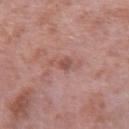notes=imaged on a skin check; not biopsied
patient=male, roughly 40 years of age
lesion size=≈4 mm
illumination=white-light
image source=total-body-photography crop, ~15 mm field of view
location=the right upper arm